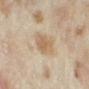image source: total-body-photography crop, ~15 mm field of view | subject: female, in their mid- to late 30s | image-analysis metrics: an area of roughly 8 mm², a shape eccentricity near 0.7, and two-axis asymmetry of about 0.2; a lesion color around L≈59 a*≈14 b*≈32 in CIELAB, a lesion–skin lightness drop of about 9, and a lesion-to-skin contrast of about 7 (normalized; higher = more distinct); a classifier nevus-likeness of about 45/100 and a detector confidence of about 100 out of 100 that the crop contains a lesion | lighting: cross-polarized | site: the arm.